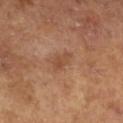<tbp_lesion>
  <biopsy_status>not biopsied; imaged during a skin examination</biopsy_status>
  <image>
    <source>total-body photography crop</source>
    <field_of_view_mm>15</field_of_view_mm>
  </image>
  <lesion_size>
    <long_diameter_mm_approx>3.0</long_diameter_mm_approx>
  </lesion_size>
  <patient>
    <sex>male</sex>
    <age_approx>65</age_approx>
  </patient>
  <lighting>cross-polarized</lighting>
  <automated_metrics>
    <area_mm2_approx>3.0</area_mm2_approx>
    <eccentricity>0.9</eccentricity>
    <cielab_L>46</cielab_L>
    <cielab_a>21</cielab_a>
    <cielab_b>31</cielab_b>
    <vs_skin_darker_L>7.0</vs_skin_darker_L>
    <vs_skin_contrast_norm>6.0</vs_skin_contrast_norm>
    <border_irregularity_0_10>3.5</border_irregularity_0_10>
    <color_variation_0_10>0.5</color_variation_0_10>
    <peripheral_color_asymmetry>0.0</peripheral_color_asymmetry>
  </automated_metrics>
</tbp_lesion>